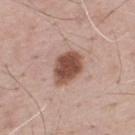Clinical impression: Part of a total-body skin-imaging series; this lesion was reviewed on a skin check and was not flagged for biopsy. Image and clinical context: This is a white-light tile. An algorithmic analysis of the crop reported peripheral color asymmetry of about 1. The analysis additionally found a classifier nevus-likeness of about 95/100 and a lesion-detection confidence of about 100/100. A roughly 15 mm field-of-view crop from a total-body skin photograph. The subject is a male aged around 70. The lesion is on the upper back.